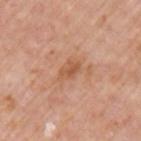| field | value |
|---|---|
| notes | catalogued during a skin exam; not biopsied |
| imaging modality | ~15 mm crop, total-body skin-cancer survey |
| automated metrics | a classifier nevus-likeness of about 0/100 and a detector confidence of about 100 out of 100 that the crop contains a lesion |
| illumination | white-light illumination |
| body site | the arm |
| size | ≈3 mm |
| subject | male, approximately 75 years of age |